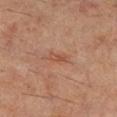Imaged during a routine full-body skin examination; the lesion was not biopsied and no histopathology is available. A 15 mm close-up tile from a total-body photography series done for melanoma screening. The lesion is on the leg. A male subject, roughly 60 years of age.